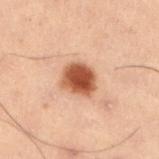No biopsy was performed on this lesion — it was imaged during a full skin examination and was not determined to be concerning.
Cropped from a whole-body photographic skin survey; the tile spans about 15 mm.
A male subject in their mid- to late 50s.
Captured under cross-polarized illumination.
The lesion is on the left lower leg.